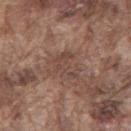No biopsy was performed on this lesion — it was imaged during a full skin examination and was not determined to be concerning.
Approximately 3.5 mm at its widest.
An algorithmic analysis of the crop reported an area of roughly 6.5 mm², an eccentricity of roughly 0.75, and a shape-asymmetry score of about 0.6 (0 = symmetric). It also reported an average lesion color of about L≈45 a*≈18 b*≈24 (CIELAB) and a lesion-to-skin contrast of about 5.5 (normalized; higher = more distinct). The software also gave an automated nevus-likeness rating near 0 out of 100.
Captured under white-light illumination.
A male subject about 75 years old.
A lesion tile, about 15 mm wide, cut from a 3D total-body photograph.
The lesion is on the mid back.